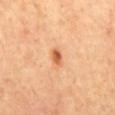The lesion was photographed on a routine skin check and not biopsied; there is no pathology result. The total-body-photography lesion software estimated a lesion color around L≈62 a*≈28 b*≈42 in CIELAB, roughly 13 lightness units darker than nearby skin, and a normalized border contrast of about 8.5. It also reported a border-irregularity rating of about 2/10 and a peripheral color-asymmetry measure near 1.5. A 15 mm close-up tile from a total-body photography series done for melanoma screening. Captured under cross-polarized illumination. The lesion is on the mid back. The lesion's longest dimension is about 2 mm. A male subject in their mid-50s.